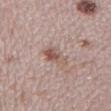Q: Is there a histopathology result?
A: imaged on a skin check; not biopsied
Q: Automated lesion metrics?
A: a classifier nevus-likeness of about 80/100 and a detector confidence of about 100 out of 100 that the crop contains a lesion
Q: Where on the body is the lesion?
A: the leg
Q: Who is the patient?
A: female, approximately 30 years of age
Q: What is the lesion's diameter?
A: about 3.5 mm
Q: What kind of image is this?
A: total-body-photography crop, ~15 mm field of view
Q: Illumination type?
A: white-light